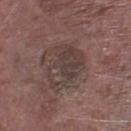On the right lower leg. Imaged with white-light lighting. The lesion's longest dimension is about 5.5 mm. A male patient, approximately 75 years of age. A close-up tile cropped from a whole-body skin photograph, about 15 mm across.